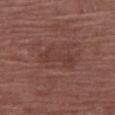Assessment:
The lesion was tiled from a total-body skin photograph and was not biopsied.
Acquisition and patient details:
The recorded lesion diameter is about 4.5 mm. A female patient, roughly 70 years of age. A 15 mm close-up extracted from a 3D total-body photography capture. On the arm. The tile uses white-light illumination.